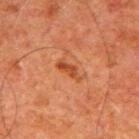This lesion was catalogued during total-body skin photography and was not selected for biopsy.
This is a cross-polarized tile.
From the mid back.
A male subject in their 80s.
About 3.5 mm across.
This image is a 15 mm lesion crop taken from a total-body photograph.